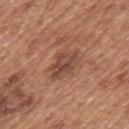Notes:
• biopsy status · catalogued during a skin exam; not biopsied
• site · the mid back
• image · total-body-photography crop, ~15 mm field of view
• diameter · about 4.5 mm
• tile lighting · white-light
• subject · male, aged around 65